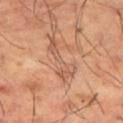Impression: No biopsy was performed on this lesion — it was imaged during a full skin examination and was not determined to be concerning. Background: An algorithmic analysis of the crop reported an eccentricity of roughly 0.95 and two-axis asymmetry of about 0.55. The analysis additionally found border irregularity of about 8 on a 0–10 scale, a color-variation rating of about 2.5/10, and a peripheral color-asymmetry measure near 1. The analysis additionally found a classifier nevus-likeness of about 0/100. The tile uses cross-polarized illumination. About 5 mm across. The lesion is located on the leg. A region of skin cropped from a whole-body photographic capture, roughly 15 mm wide. The subject is a male in their mid- to late 60s.